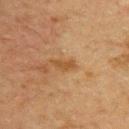{"patient": {"sex": "female", "age_approx": 55}, "image": {"source": "total-body photography crop", "field_of_view_mm": 15}, "site": "upper back"}